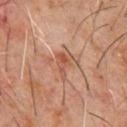Notes:
- image source · ~15 mm tile from a whole-body skin photo
- subject · male, aged approximately 60
- lighting · cross-polarized
- lesion diameter · about 3.5 mm
- anatomic site · the chest